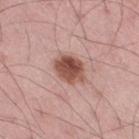The lesion is located on the left thigh. Imaged with white-light lighting. A lesion tile, about 15 mm wide, cut from a 3D total-body photograph. The total-body-photography lesion software estimated two-axis asymmetry of about 0.15. The analysis additionally found a mean CIELAB color near L≈51 a*≈23 b*≈26, about 15 CIELAB-L* units darker than the surrounding skin, and a normalized border contrast of about 10.5. It also reported a border-irregularity index near 1.5/10, a within-lesion color-variation index near 6/10, and a peripheral color-asymmetry measure near 2.5. The subject is a male approximately 45 years of age. The lesion's longest dimension is about 4 mm.